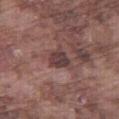Notes:
– follow-up: catalogued during a skin exam; not biopsied
– acquisition: 15 mm crop, total-body photography
– site: the right thigh
– patient: male, aged approximately 75
– automated lesion analysis: a lesion–skin lightness drop of about 10 and a normalized lesion–skin contrast near 9; an automated nevus-likeness rating near 0 out of 100